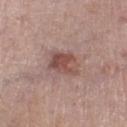Q: Was a biopsy performed?
A: catalogued during a skin exam; not biopsied
Q: Patient demographics?
A: male, aged approximately 85 or older
Q: How large is the lesion?
A: ≈3.5 mm
Q: What did automated image analysis measure?
A: a border-irregularity index near 2.5/10, a color-variation rating of about 5.5/10, and radial color variation of about 2
Q: Illumination type?
A: white-light illumination
Q: What is the imaging modality?
A: ~15 mm crop, total-body skin-cancer survey
Q: Lesion location?
A: the left lower leg